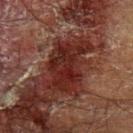{"biopsy_status": "not biopsied; imaged during a skin examination", "lesion_size": {"long_diameter_mm_approx": 7.0}, "patient": {"sex": "male", "age_approx": 70}, "lighting": "cross-polarized", "image": {"source": "total-body photography crop", "field_of_view_mm": 15}, "site": "left upper arm"}